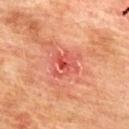<record>
  <biopsy_status>not biopsied; imaged during a skin examination</biopsy_status>
  <site>mid back</site>
  <image>
    <source>total-body photography crop</source>
    <field_of_view_mm>15</field_of_view_mm>
  </image>
  <automated_metrics>
    <border_irregularity_0_10>3.5</border_irregularity_0_10>
    <color_variation_0_10>6.5</color_variation_0_10>
    <peripheral_color_asymmetry>2.0</peripheral_color_asymmetry>
    <lesion_detection_confidence_0_100>100</lesion_detection_confidence_0_100>
  </automated_metrics>
  <patient>
    <sex>male</sex>
    <age_approx>75</age_approx>
  </patient>
  <lesion_size>
    <long_diameter_mm_approx>3.5</long_diameter_mm_approx>
  </lesion_size>
  <lighting>cross-polarized</lighting>
</record>